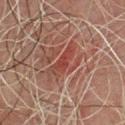follow-up: no biopsy performed (imaged during a skin exam).